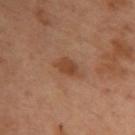workup = total-body-photography surveillance lesion; no biopsy
location = the upper back
TBP lesion metrics = a lesion color around L≈36 a*≈18 b*≈27 in CIELAB, a lesion–skin lightness drop of about 7, and a normalized lesion–skin contrast near 7; a nevus-likeness score of about 55/100
image = ~15 mm crop, total-body skin-cancer survey
patient = female, aged approximately 55
size = about 3.5 mm